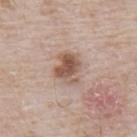Assessment: Part of a total-body skin-imaging series; this lesion was reviewed on a skin check and was not flagged for biopsy. Background: A roughly 15 mm field-of-view crop from a total-body skin photograph. The patient is a male aged 78 to 82. Located on the abdomen. The lesion's longest dimension is about 4 mm. The total-body-photography lesion software estimated an automated nevus-likeness rating near 70 out of 100 and a lesion-detection confidence of about 100/100.